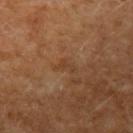{"biopsy_status": "not biopsied; imaged during a skin examination", "lesion_size": {"long_diameter_mm_approx": 2.5}, "patient": {"sex": "male", "age_approx": 55}, "lighting": "cross-polarized", "automated_metrics": {"border_irregularity_0_10": 5.5, "color_variation_0_10": 0.0, "peripheral_color_asymmetry": 0.0, "nevus_likeness_0_100": 0, "lesion_detection_confidence_0_100": 100}, "image": {"source": "total-body photography crop", "field_of_view_mm": 15}, "site": "left upper arm"}